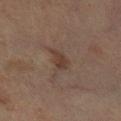Findings:
– biopsy status — total-body-photography surveillance lesion; no biopsy
– anatomic site — the leg
– imaging modality — total-body-photography crop, ~15 mm field of view
– patient — male, roughly 65 years of age